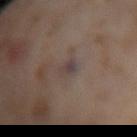Imaged during a routine full-body skin examination; the lesion was not biopsied and no histopathology is available.
Measured at roughly 2.5 mm in maximum diameter.
A lesion tile, about 15 mm wide, cut from a 3D total-body photograph.
Located on the left thigh.
The patient is a female approximately 55 years of age.
Automated image analysis of the tile measured a detector confidence of about 70 out of 100 that the crop contains a lesion.
Imaged with cross-polarized lighting.